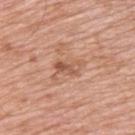workup = total-body-photography surveillance lesion; no biopsy | image source = total-body-photography crop, ~15 mm field of view | subject = female, approximately 45 years of age | anatomic site = the upper back.